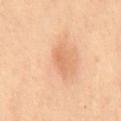Imaged during a routine full-body skin examination; the lesion was not biopsied and no histopathology is available. Measured at roughly 1.5 mm in maximum diameter. This image is a 15 mm lesion crop taken from a total-body photograph. Captured under cross-polarized illumination. On the mid back. A male subject, about 55 years old. The lesion-visualizer software estimated a footprint of about 1.5 mm² and an outline eccentricity of about 0.8 (0 = round, 1 = elongated). The software also gave an average lesion color of about L≈66 a*≈25 b*≈37 (CIELAB) and a normalized lesion–skin contrast near 4. And it measured border irregularity of about 2.5 on a 0–10 scale, a within-lesion color-variation index near 0/10, and peripheral color asymmetry of about 0.